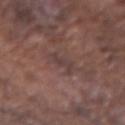workup = imaged on a skin check; not biopsied
diameter = ~2.5 mm (longest diameter)
site = the left forearm
illumination = white-light
patient = male, in their mid-70s
acquisition = 15 mm crop, total-body photography
automated metrics = a footprint of about 3.5 mm², an eccentricity of roughly 0.8, and two-axis asymmetry of about 0.35; about 6 CIELAB-L* units darker than the surrounding skin and a normalized lesion–skin contrast near 5.5; peripheral color asymmetry of about 0; an automated nevus-likeness rating near 0 out of 100